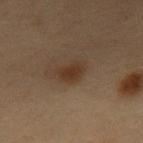Assessment: Part of a total-body skin-imaging series; this lesion was reviewed on a skin check and was not flagged for biopsy. Acquisition and patient details: A male patient, aged 53–57. Approximately 3 mm at its widest. This image is a 15 mm lesion crop taken from a total-body photograph. Automated tile analysis of the lesion measured an average lesion color of about L≈27 a*≈13 b*≈23 (CIELAB), roughly 6 lightness units darker than nearby skin, and a normalized border contrast of about 7.5. The lesion is located on the abdomen.